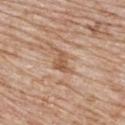Part of a total-body skin-imaging series; this lesion was reviewed on a skin check and was not flagged for biopsy. A female patient, aged approximately 85 or older. The tile uses white-light illumination. A 15 mm close-up tile from a total-body photography series done for melanoma screening. Longest diameter approximately 3 mm. On the upper back.